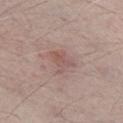Q: Was a biopsy performed?
A: catalogued during a skin exam; not biopsied
Q: How was this image acquired?
A: total-body-photography crop, ~15 mm field of view
Q: Where on the body is the lesion?
A: the right lower leg
Q: How was the tile lit?
A: white-light
Q: Lesion size?
A: ~3 mm (longest diameter)
Q: Who is the patient?
A: male, about 65 years old